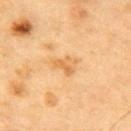| key | value |
|---|---|
| notes | total-body-photography surveillance lesion; no biopsy |
| tile lighting | cross-polarized |
| location | the chest |
| subject | male, in their mid- to late 80s or older |
| imaging modality | ~15 mm crop, total-body skin-cancer survey |
| lesion diameter | about 3.5 mm |